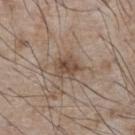Assessment:
The lesion was photographed on a routine skin check and not biopsied; there is no pathology result.
Image and clinical context:
This is a white-light tile. The lesion is on the chest. Approximately 3 mm at its widest. A male patient in their mid- to late 70s. Cropped from a whole-body photographic skin survey; the tile spans about 15 mm.